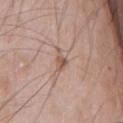Notes:
– notes — imaged on a skin check; not biopsied
– body site — the chest
– lesion diameter — about 3 mm
– imaging modality — total-body-photography crop, ~15 mm field of view
– image-analysis metrics — a mean CIELAB color near L≈55 a*≈18 b*≈26 and about 10 CIELAB-L* units darker than the surrounding skin; an automated nevus-likeness rating near 0 out of 100 and a detector confidence of about 95 out of 100 that the crop contains a lesion
– subject — male, approximately 65 years of age